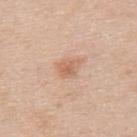Part of a total-body skin-imaging series; this lesion was reviewed on a skin check and was not flagged for biopsy. A region of skin cropped from a whole-body photographic capture, roughly 15 mm wide. Longest diameter approximately 3 mm. Captured under white-light illumination. On the upper back. A male subject about 35 years old.